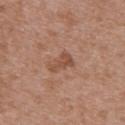notes — no biopsy performed (imaged during a skin exam)
imaging modality — ~15 mm crop, total-body skin-cancer survey
size — ≈3 mm
TBP lesion metrics — a shape eccentricity near 0.85 and a shape-asymmetry score of about 0.45 (0 = symmetric); a border-irregularity rating of about 4.5/10 and a peripheral color-asymmetry measure near 0.5
patient — female, aged approximately 30
location — the back
illumination — white-light illumination